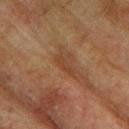The lesion was photographed on a routine skin check and not biopsied; there is no pathology result. The lesion-visualizer software estimated a footprint of about 2.5 mm², a shape eccentricity near 0.95, and a shape-asymmetry score of about 0.25 (0 = symmetric). The patient is a male aged approximately 75. About 3 mm across. This image is a 15 mm lesion crop taken from a total-body photograph. On the upper back.